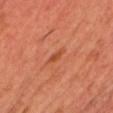Clinical impression: Imaged during a routine full-body skin examination; the lesion was not biopsied and no histopathology is available. Background: A male subject aged approximately 45. Cropped from a whole-body photographic skin survey; the tile spans about 15 mm. This is a cross-polarized tile. On the chest.